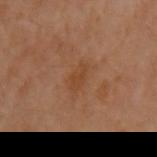Impression:
Part of a total-body skin-imaging series; this lesion was reviewed on a skin check and was not flagged for biopsy.
Background:
Automated tile analysis of the lesion measured a footprint of about 4 mm², an outline eccentricity of about 0.8 (0 = round, 1 = elongated), and a shape-asymmetry score of about 0.25 (0 = symmetric). Captured under cross-polarized illumination. The subject is a female roughly 60 years of age. The lesion is located on the left arm. A lesion tile, about 15 mm wide, cut from a 3D total-body photograph.